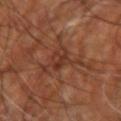Recorded during total-body skin imaging; not selected for excision or biopsy. A lesion tile, about 15 mm wide, cut from a 3D total-body photograph. The patient is aged approximately 65. From the right upper arm. Captured under cross-polarized illumination. Longest diameter approximately 5.5 mm.